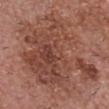biopsy status = imaged on a skin check; not biopsied | lighting = white-light | automated lesion analysis = an average lesion color of about L≈46 a*≈23 b*≈27 (CIELAB) and about 9 CIELAB-L* units darker than the surrounding skin; a nevus-likeness score of about 0/100 | subject = male, roughly 80 years of age | imaging modality = total-body-photography crop, ~15 mm field of view | body site = the head or neck.